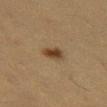No biopsy was performed on this lesion — it was imaged during a full skin examination and was not determined to be concerning. From the left thigh. A female subject approximately 40 years of age. The tile uses cross-polarized illumination. A roughly 15 mm field-of-view crop from a total-body skin photograph. Measured at roughly 3 mm in maximum diameter.